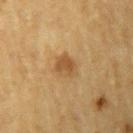<lesion>
<biopsy_status>not biopsied; imaged during a skin examination</biopsy_status>
<image>
  <source>total-body photography crop</source>
  <field_of_view_mm>15</field_of_view_mm>
</image>
<lesion_size>
  <long_diameter_mm_approx>3.0</long_diameter_mm_approx>
</lesion_size>
<lighting>cross-polarized</lighting>
<patient>
  <sex>male</sex>
  <age_approx>85+</age_approx>
</patient>
<site>left upper arm</site>
</lesion>